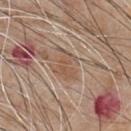Impression: The lesion was photographed on a routine skin check and not biopsied; there is no pathology result. Image and clinical context: Automated image analysis of the tile measured a border-irregularity rating of about 7.5/10, a within-lesion color-variation index near 2.5/10, and peripheral color asymmetry of about 1. The software also gave a classifier nevus-likeness of about 50/100 and lesion-presence confidence of about 80/100. Approximately 4 mm at its widest. The lesion is located on the chest. A 15 mm crop from a total-body photograph taken for skin-cancer surveillance. The tile uses white-light illumination. A male subject aged 63–67.